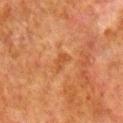Part of a total-body skin-imaging series; this lesion was reviewed on a skin check and was not flagged for biopsy. The lesion is on the right lower leg. The subject is a male aged 78–82. A roughly 15 mm field-of-view crop from a total-body skin photograph.